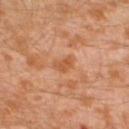Clinical impression: Imaged during a routine full-body skin examination; the lesion was not biopsied and no histopathology is available. Acquisition and patient details: From the left lower leg. Captured under cross-polarized illumination. A region of skin cropped from a whole-body photographic capture, roughly 15 mm wide. The total-body-photography lesion software estimated about 8 CIELAB-L* units darker than the surrounding skin. The analysis additionally found a color-variation rating of about 2/10 and a peripheral color-asymmetry measure near 1. It also reported an automated nevus-likeness rating near 0 out of 100. The recorded lesion diameter is about 2.5 mm. The subject is a male about 30 years old.